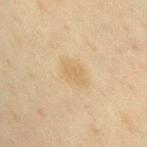Clinical impression: No biopsy was performed on this lesion — it was imaged during a full skin examination and was not determined to be concerning. Acquisition and patient details: The tile uses cross-polarized illumination. Located on the chest. Measured at roughly 3 mm in maximum diameter. Cropped from a total-body skin-imaging series; the visible field is about 15 mm. A female patient in their 40s.